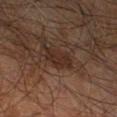image source — total-body-photography crop, ~15 mm field of view; anatomic site — the leg; subject — male, aged approximately 60.